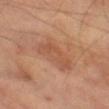{"biopsy_status": "not biopsied; imaged during a skin examination", "lighting": "cross-polarized", "automated_metrics": {"area_mm2_approx": 13.0, "eccentricity": 0.9, "shape_asymmetry": 0.25, "border_irregularity_0_10": 3.5, "color_variation_0_10": 3.0, "peripheral_color_asymmetry": 1.0}, "image": {"source": "total-body photography crop", "field_of_view_mm": 15}, "lesion_size": {"long_diameter_mm_approx": 6.0}, "patient": {"sex": "male", "age_approx": 70}, "site": "left thigh"}